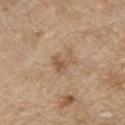<tbp_lesion>
<biopsy_status>not biopsied; imaged during a skin examination</biopsy_status>
<image>
  <source>total-body photography crop</source>
  <field_of_view_mm>15</field_of_view_mm>
</image>
<patient>
  <sex>male</sex>
  <age_approx>70</age_approx>
</patient>
<site>chest</site>
</tbp_lesion>